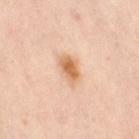The lesion was tiled from a total-body skin photograph and was not biopsied. About 4 mm across. Cropped from a whole-body photographic skin survey; the tile spans about 15 mm. The subject is a female about 40 years old. Imaged with cross-polarized lighting. The lesion is on the left leg.